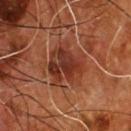– follow-up — imaged on a skin check; not biopsied
– subject — male, in their mid- to late 50s
– image-analysis metrics — a lesion area of about 15 mm² and a shape-asymmetry score of about 0.35 (0 = symmetric); a border-irregularity index near 4/10, a within-lesion color-variation index near 6/10, and peripheral color asymmetry of about 2; a detector confidence of about 100 out of 100 that the crop contains a lesion
– acquisition — 15 mm crop, total-body photography
– illumination — cross-polarized
– site — the front of the torso